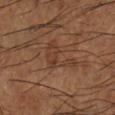{
  "biopsy_status": "not biopsied; imaged during a skin examination",
  "lesion_size": {
    "long_diameter_mm_approx": 5.0
  },
  "site": "left lower leg",
  "patient": {
    "sex": "male",
    "age_approx": 65
  },
  "automated_metrics": {
    "area_mm2_approx": 8.0,
    "shape_asymmetry": 0.65,
    "cielab_L": 38,
    "cielab_a": 19,
    "cielab_b": 28,
    "vs_skin_darker_L": 6.0,
    "vs_skin_contrast_norm": 5.0,
    "border_irregularity_0_10": 8.0,
    "color_variation_0_10": 2.5,
    "peripheral_color_asymmetry": 1.0,
    "nevus_likeness_0_100": 0,
    "lesion_detection_confidence_0_100": 70
  },
  "image": {
    "source": "total-body photography crop",
    "field_of_view_mm": 15
  }
}